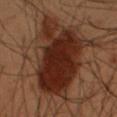Recorded during total-body skin imaging; not selected for excision or biopsy. A male patient, aged 53–57. Cropped from a total-body skin-imaging series; the visible field is about 15 mm. Measured at roughly 9.5 mm in maximum diameter. Captured under cross-polarized illumination. Located on the right forearm. An algorithmic analysis of the crop reported a lesion area of about 45 mm², an eccentricity of roughly 0.7, and two-axis asymmetry of about 0.3. And it measured an average lesion color of about L≈21 a*≈18 b*≈22 (CIELAB) and roughly 11 lightness units darker than nearby skin. And it measured border irregularity of about 3.5 on a 0–10 scale, a within-lesion color-variation index near 4.5/10, and a peripheral color-asymmetry measure near 1.5.